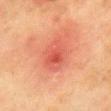Q: Was a biopsy performed?
A: total-body-photography surveillance lesion; no biopsy
Q: How was this image acquired?
A: total-body-photography crop, ~15 mm field of view
Q: Lesion location?
A: the chest
Q: Patient demographics?
A: female, roughly 50 years of age